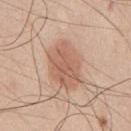Recorded during total-body skin imaging; not selected for excision or biopsy. Located on the chest. A male patient roughly 50 years of age. An algorithmic analysis of the crop reported roughly 10 lightness units darker than nearby skin and a lesion-to-skin contrast of about 6.5 (normalized; higher = more distinct). The analysis additionally found a nevus-likeness score of about 95/100 and lesion-presence confidence of about 100/100. About 6 mm across. A 15 mm close-up extracted from a 3D total-body photography capture.